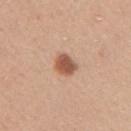Clinical impression:
Captured during whole-body skin photography for melanoma surveillance; the lesion was not biopsied.
Image and clinical context:
A female patient, roughly 45 years of age. Automated image analysis of the tile measured a footprint of about 5 mm², an outline eccentricity of about 0.6 (0 = round, 1 = elongated), and a shape-asymmetry score of about 0.15 (0 = symmetric). It also reported a peripheral color-asymmetry measure near 0.5. The analysis additionally found a nevus-likeness score of about 100/100 and lesion-presence confidence of about 100/100. The recorded lesion diameter is about 2.5 mm. The lesion is on the right upper arm. A close-up tile cropped from a whole-body skin photograph, about 15 mm across. The tile uses white-light illumination.